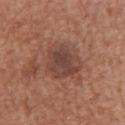Notes:
* notes · total-body-photography surveillance lesion; no biopsy
* imaging modality · 15 mm crop, total-body photography
* anatomic site · the left upper arm
* subject · male, approximately 65 years of age